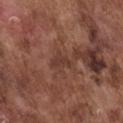Clinical summary: A male patient, aged 73 to 77. This is a white-light tile. From the chest. This image is a 15 mm lesion crop taken from a total-body photograph.